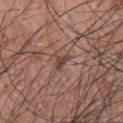Assessment:
The lesion was photographed on a routine skin check and not biopsied; there is no pathology result.
Context:
A male subject, aged 68 to 72. A roughly 15 mm field-of-view crop from a total-body skin photograph. From the back.